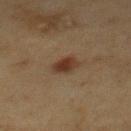Clinical impression:
No biopsy was performed on this lesion — it was imaged during a full skin examination and was not determined to be concerning.
Context:
A region of skin cropped from a whole-body photographic capture, roughly 15 mm wide. This is a cross-polarized tile. On the back. The lesion's longest dimension is about 3 mm. The subject is a male roughly 60 years of age.